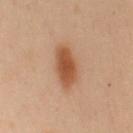Clinical impression: This lesion was catalogued during total-body skin photography and was not selected for biopsy. Clinical summary: From the mid back. The recorded lesion diameter is about 5 mm. This image is a 15 mm lesion crop taken from a total-body photograph. A female subject approximately 50 years of age. Captured under cross-polarized illumination.